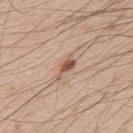{"biopsy_status": "not biopsied; imaged during a skin examination", "site": "upper back", "image": {"source": "total-body photography crop", "field_of_view_mm": 15}, "patient": {"sex": "male", "age_approx": 50}, "automated_metrics": {"vs_skin_darker_L": 12.0, "lesion_detection_confidence_0_100": 100}}